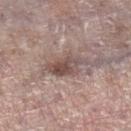subject — female, approximately 85 years of age or older
body site — the right lower leg
acquisition — ~15 mm crop, total-body skin-cancer survey